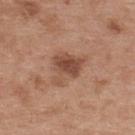| field | value |
|---|---|
| TBP lesion metrics | a lesion–skin lightness drop of about 11 and a lesion-to-skin contrast of about 7.5 (normalized; higher = more distinct); border irregularity of about 6 on a 0–10 scale and peripheral color asymmetry of about 1; a classifier nevus-likeness of about 55/100 and lesion-presence confidence of about 100/100 |
| location | the back |
| image | 15 mm crop, total-body photography |
| patient | female, aged approximately 40 |
| lesion diameter | about 5 mm |
| tile lighting | white-light illumination |